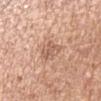The lesion-visualizer software estimated border irregularity of about 3 on a 0–10 scale, a within-lesion color-variation index near 3/10, and peripheral color asymmetry of about 1. And it measured a classifier nevus-likeness of about 0/100 and a detector confidence of about 100 out of 100 that the crop contains a lesion.
A lesion tile, about 15 mm wide, cut from a 3D total-body photograph.
A male patient roughly 65 years of age.
Captured under white-light illumination.
Longest diameter approximately 3.5 mm.
Located on the right upper arm.